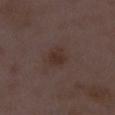Impression: The lesion was photographed on a routine skin check and not biopsied; there is no pathology result. Image and clinical context: From the right lower leg. The lesion-visualizer software estimated an area of roughly 4.5 mm², an outline eccentricity of about 0.55 (0 = round, 1 = elongated), and two-axis asymmetry of about 0.3. The analysis additionally found a mean CIELAB color near L≈30 a*≈15 b*≈21, a lesion–skin lightness drop of about 6, and a normalized lesion–skin contrast near 7. It also reported a border-irregularity index near 2.5/10, a within-lesion color-variation index near 2/10, and radial color variation of about 0.5. A female subject, in their 30s. A close-up tile cropped from a whole-body skin photograph, about 15 mm across.